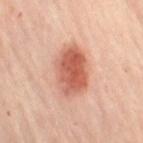Captured during whole-body skin photography for melanoma surveillance; the lesion was not biopsied.
Automated tile analysis of the lesion measured a lesion color around L≈59 a*≈28 b*≈31 in CIELAB, a lesion–skin lightness drop of about 14, and a normalized lesion–skin contrast near 9. The analysis additionally found an automated nevus-likeness rating near 100 out of 100 and lesion-presence confidence of about 100/100.
A female patient, aged around 60.
This image is a 15 mm lesion crop taken from a total-body photograph.
The lesion is on the right thigh.
This is a cross-polarized tile.
Measured at roughly 6 mm in maximum diameter.